On the head or neck; a lesion tile, about 15 mm wide, cut from a 3D total-body photograph; the subject is a male aged 63–67:
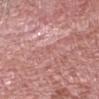Context: This is a white-light tile. The recorded lesion diameter is about 1.5 mm. The lesion-visualizer software estimated a lesion area of about 1 mm², an outline eccentricity of about 0.9 (0 = round, 1 = elongated), and two-axis asymmetry of about 0.45. And it measured a border-irregularity index near 3.5/10, a color-variation rating of about 0/10, and peripheral color asymmetry of about 0. Conclusion: Histopathology of the biopsied lesion showed a nodular basal cell carcinoma — a malignancy.